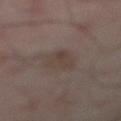- workup · imaged on a skin check; not biopsied
- patient · male, aged around 50
- body site · the front of the torso
- acquisition · ~15 mm crop, total-body skin-cancer survey
- illumination · cross-polarized illumination
- automated metrics · a footprint of about 7 mm² and two-axis asymmetry of about 0.25